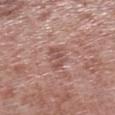Clinical impression: Part of a total-body skin-imaging series; this lesion was reviewed on a skin check and was not flagged for biopsy. Image and clinical context: From the left lower leg. A roughly 15 mm field-of-view crop from a total-body skin photograph. A male patient, about 55 years old. Longest diameter approximately 3 mm. Imaged with white-light lighting.